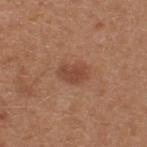Part of a total-body skin-imaging series; this lesion was reviewed on a skin check and was not flagged for biopsy.
A region of skin cropped from a whole-body photographic capture, roughly 15 mm wide.
The recorded lesion diameter is about 3.5 mm.
A male patient approximately 65 years of age.
Captured under white-light illumination.
An algorithmic analysis of the crop reported a nevus-likeness score of about 70/100 and a lesion-detection confidence of about 100/100.
Located on the upper back.